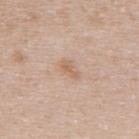Findings:
- follow-up — catalogued during a skin exam; not biopsied
- anatomic site — the upper back
- image source — total-body-photography crop, ~15 mm field of view
- patient — female, about 40 years old